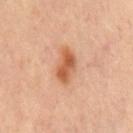Impression: Captured during whole-body skin photography for melanoma surveillance; the lesion was not biopsied. Clinical summary: The lesion's longest dimension is about 4.5 mm. A lesion tile, about 15 mm wide, cut from a 3D total-body photograph. On the chest. Captured under cross-polarized illumination. A male subject, about 65 years old. Automated tile analysis of the lesion measured two-axis asymmetry of about 0.25.